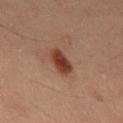| feature | finding |
|---|---|
| biopsy status | total-body-photography surveillance lesion; no biopsy |
| subject | male, in their 60s |
| lighting | cross-polarized |
| body site | the back |
| image source | 15 mm crop, total-body photography |
| size | ~3 mm (longest diameter) |